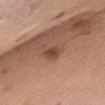Recorded during total-body skin imaging; not selected for excision or biopsy. A 15 mm close-up extracted from a 3D total-body photography capture. The lesion is located on the chest. The subject is a female in their mid-50s.